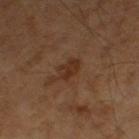| field | value |
|---|---|
| image source | total-body-photography crop, ~15 mm field of view |
| subject | male, aged around 60 |
| location | the left thigh |
| illumination | cross-polarized illumination |
| automated metrics | a footprint of about 5 mm², an outline eccentricity of about 0.8 (0 = round, 1 = elongated), and two-axis asymmetry of about 0.25; a within-lesion color-variation index near 2.5/10 and radial color variation of about 1; a classifier nevus-likeness of about 10/100 and a lesion-detection confidence of about 100/100 |
| diameter | ≈3 mm |